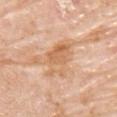Recorded during total-body skin imaging; not selected for excision or biopsy.
The total-body-photography lesion software estimated an outline eccentricity of about 0.85 (0 = round, 1 = elongated) and two-axis asymmetry of about 0.3. It also reported a mean CIELAB color near L≈65 a*≈22 b*≈37, a lesion–skin lightness drop of about 9, and a normalized border contrast of about 6.5.
A male subject, about 75 years old.
On the chest.
A roughly 15 mm field-of-view crop from a total-body skin photograph.
The lesion's longest dimension is about 5.5 mm.